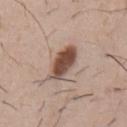{"biopsy_status": "not biopsied; imaged during a skin examination", "patient": {"sex": "male", "age_approx": 50}, "site": "chest", "lesion_size": {"long_diameter_mm_approx": 5.0}, "image": {"source": "total-body photography crop", "field_of_view_mm": 15}, "lighting": "white-light"}